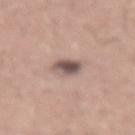Context:
This is a white-light tile. From the lower back. Measured at roughly 2.5 mm in maximum diameter. An algorithmic analysis of the crop reported a footprint of about 5.5 mm² and a shape eccentricity near 0.35. A lesion tile, about 15 mm wide, cut from a 3D total-body photograph. A male subject in their mid- to late 40s.